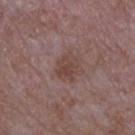Assessment:
Imaged during a routine full-body skin examination; the lesion was not biopsied and no histopathology is available.